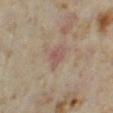Clinical impression: The lesion was tiled from a total-body skin photograph and was not biopsied. Context: The lesion is located on the left thigh. Approximately 2.5 mm at its widest. A region of skin cropped from a whole-body photographic capture, roughly 15 mm wide. Imaged with cross-polarized lighting. A female subject, aged 33 to 37.